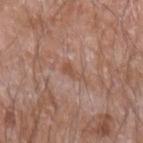No biopsy was performed on this lesion — it was imaged during a full skin examination and was not determined to be concerning. The subject is a male roughly 60 years of age. The lesion is on the arm. A 15 mm close-up tile from a total-body photography series done for melanoma screening. Automated tile analysis of the lesion measured an area of roughly 2.5 mm² and an eccentricity of roughly 0.9. It also reported an automated nevus-likeness rating near 0 out of 100 and a lesion-detection confidence of about 100/100. Longest diameter approximately 2.5 mm.